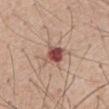<record>
<biopsy_status>not biopsied; imaged during a skin examination</biopsy_status>
<automated_metrics>
  <cielab_L>50</cielab_L>
  <cielab_a>25</cielab_a>
  <cielab_b>24</cielab_b>
  <vs_skin_darker_L>16.0</vs_skin_darker_L>
  <vs_skin_contrast_norm>11.0</vs_skin_contrast_norm>
  <nevus_likeness_0_100>5</nevus_likeness_0_100>
  <lesion_detection_confidence_0_100>100</lesion_detection_confidence_0_100>
</automated_metrics>
<lesion_size>
  <long_diameter_mm_approx>3.0</long_diameter_mm_approx>
</lesion_size>
<patient>
  <sex>male</sex>
  <age_approx>60</age_approx>
</patient>
<image>
  <source>total-body photography crop</source>
  <field_of_view_mm>15</field_of_view_mm>
</image>
<site>chest</site>
</record>